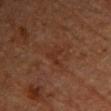Case summary:
• follow-up: total-body-photography surveillance lesion; no biopsy
• diameter: ≈3 mm
• TBP lesion metrics: a footprint of about 3.5 mm², a shape eccentricity near 0.75, and two-axis asymmetry of about 0.65; a lesion color around L≈25 a*≈19 b*≈24 in CIELAB
• patient: female, in their 70s
• anatomic site: the right forearm
• lighting: cross-polarized
• acquisition: ~15 mm crop, total-body skin-cancer survey